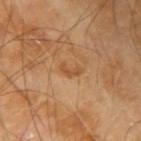<lesion>
  <biopsy_status>not biopsied; imaged during a skin examination</biopsy_status>
  <patient>
    <sex>male</sex>
    <age_approx>65</age_approx>
  </patient>
  <lighting>cross-polarized</lighting>
  <lesion_size>
    <long_diameter_mm_approx>2.5</long_diameter_mm_approx>
  </lesion_size>
  <automated_metrics>
    <cielab_L>52</cielab_L>
    <cielab_a>23</cielab_a>
    <cielab_b>39</cielab_b>
    <vs_skin_darker_L>8.0</vs_skin_darker_L>
    <vs_skin_contrast_norm>6.0</vs_skin_contrast_norm>
    <nevus_likeness_0_100>0</nevus_likeness_0_100>
    <lesion_detection_confidence_0_100>100</lesion_detection_confidence_0_100>
  </automated_metrics>
  <image>
    <source>total-body photography crop</source>
    <field_of_view_mm>15</field_of_view_mm>
  </image>
  <site>right upper arm</site>
</lesion>